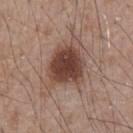Assessment:
The lesion was tiled from a total-body skin photograph and was not biopsied.
Acquisition and patient details:
Imaged with white-light lighting. The recorded lesion diameter is about 5 mm. The lesion is located on the chest. Automated tile analysis of the lesion measured an area of roughly 16 mm², an outline eccentricity of about 0.35 (0 = round, 1 = elongated), and a symmetry-axis asymmetry near 0.25. And it measured a mean CIELAB color near L≈42 a*≈19 b*≈24 and a normalized lesion–skin contrast near 11. And it measured an automated nevus-likeness rating near 65 out of 100 and lesion-presence confidence of about 100/100. A male subject, in their mid-60s. A 15 mm close-up tile from a total-body photography series done for melanoma screening.